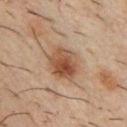Background: Captured under cross-polarized illumination. A male patient, aged 33–37. The lesion is on the chest. Cropped from a whole-body photographic skin survey; the tile spans about 15 mm. The lesion's longest dimension is about 4.5 mm. Automated image analysis of the tile measured a lesion color around L≈46 a*≈19 b*≈29 in CIELAB, roughly 11 lightness units darker than nearby skin, and a lesion-to-skin contrast of about 8.5 (normalized; higher = more distinct). The analysis additionally found border irregularity of about 2 on a 0–10 scale, a within-lesion color-variation index near 6.5/10, and peripheral color asymmetry of about 2.